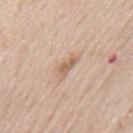Q: Was a biopsy performed?
A: total-body-photography surveillance lesion; no biopsy
Q: What are the patient's age and sex?
A: male, aged 63 to 67
Q: Lesion location?
A: the mid back
Q: What is the imaging modality?
A: 15 mm crop, total-body photography